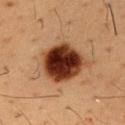Captured during whole-body skin photography for melanoma surveillance; the lesion was not biopsied. Automated tile analysis of the lesion measured an outline eccentricity of about 0.55 (0 = round, 1 = elongated) and two-axis asymmetry of about 0.15. It also reported border irregularity of about 1.5 on a 0–10 scale, internal color variation of about 8.5 on a 0–10 scale, and a peripheral color-asymmetry measure near 2.5. A male patient, about 55 years old. Measured at roughly 5.5 mm in maximum diameter. This is a cross-polarized tile. A region of skin cropped from a whole-body photographic capture, roughly 15 mm wide. The lesion is on the chest.